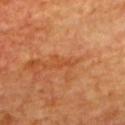<record>
  <biopsy_status>not biopsied; imaged during a skin examination</biopsy_status>
  <site>back</site>
  <lighting>cross-polarized</lighting>
  <lesion_size>
    <long_diameter_mm_approx>2.5</long_diameter_mm_approx>
  </lesion_size>
  <patient>
    <sex>male</sex>
    <age_approx>65</age_approx>
  </patient>
  <image>
    <source>total-body photography crop</source>
    <field_of_view_mm>15</field_of_view_mm>
  </image>
</record>